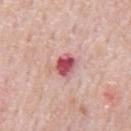Impression: The lesion was photographed on a routine skin check and not biopsied; there is no pathology result. Background: A male subject roughly 65 years of age. Located on the back. This image is a 15 mm lesion crop taken from a total-body photograph.